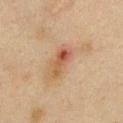Clinical impression: No biopsy was performed on this lesion — it was imaged during a full skin examination and was not determined to be concerning. Image and clinical context: Located on the chest. A lesion tile, about 15 mm wide, cut from a 3D total-body photograph. The tile uses cross-polarized illumination. The lesion's longest dimension is about 4 mm. The total-body-photography lesion software estimated a lesion area of about 5.5 mm². The analysis additionally found a detector confidence of about 100 out of 100 that the crop contains a lesion. A female patient, roughly 40 years of age.